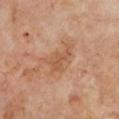workup = catalogued during a skin exam; not biopsied | lighting = cross-polarized illumination | diameter = ~4 mm (longest diameter) | subject = female, aged 58–62 | location = the chest | imaging modality = ~15 mm crop, total-body skin-cancer survey.